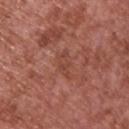Case summary:
* notes: catalogued during a skin exam; not biopsied
* image: ~15 mm crop, total-body skin-cancer survey
* subject: male, in their mid-60s
* location: the chest